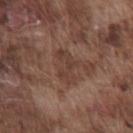Captured during whole-body skin photography for melanoma surveillance; the lesion was not biopsied. From the chest. The patient is a male aged 73 to 77. A 15 mm crop from a total-body photograph taken for skin-cancer surveillance. The lesion's longest dimension is about 4 mm.